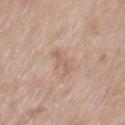Notes:
– notes: total-body-photography surveillance lesion; no biopsy
– patient: female, roughly 75 years of age
– acquisition: ~15 mm crop, total-body skin-cancer survey
– anatomic site: the left thigh